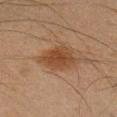This lesion was catalogued during total-body skin photography and was not selected for biopsy.
A roughly 15 mm field-of-view crop from a total-body skin photograph.
This is a cross-polarized tile.
The subject is a male approximately 35 years of age.
On the left forearm.